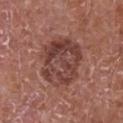This lesion was catalogued during total-body skin photography and was not selected for biopsy.